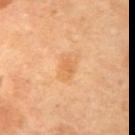Q: Was a biopsy performed?
A: imaged on a skin check; not biopsied
Q: Lesion size?
A: ≈2.5 mm
Q: Who is the patient?
A: female, aged approximately 70
Q: What did automated image analysis measure?
A: a shape-asymmetry score of about 0.2 (0 = symmetric)
Q: Lesion location?
A: the right upper arm
Q: How was this image acquired?
A: total-body-photography crop, ~15 mm field of view